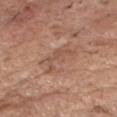follow-up: no biopsy performed (imaged during a skin exam); site: the chest; image-analysis metrics: border irregularity of about 6 on a 0–10 scale, a within-lesion color-variation index near 2/10, and peripheral color asymmetry of about 0.5; lesion diameter: ~4.5 mm (longest diameter); illumination: white-light illumination; image: 15 mm crop, total-body photography; subject: male, in their 80s.